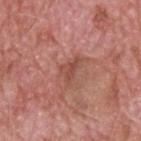Captured during whole-body skin photography for melanoma surveillance; the lesion was not biopsied.
The lesion's longest dimension is about 3.5 mm.
From the back.
An algorithmic analysis of the crop reported a border-irregularity index near 6.5/10. The analysis additionally found an automated nevus-likeness rating near 0 out of 100 and lesion-presence confidence of about 90/100.
Imaged with white-light lighting.
A 15 mm crop from a total-body photograph taken for skin-cancer surveillance.
A male patient, roughly 60 years of age.